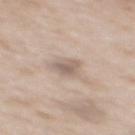Part of a total-body skin-imaging series; this lesion was reviewed on a skin check and was not flagged for biopsy. A male subject, aged 63–67. A roughly 15 mm field-of-view crop from a total-body skin photograph. The lesion's longest dimension is about 2.5 mm. On the mid back. Captured under white-light illumination.